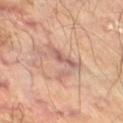Assessment:
This lesion was catalogued during total-body skin photography and was not selected for biopsy.
Background:
Cropped from a whole-body photographic skin survey; the tile spans about 15 mm. The lesion is located on the leg. A male patient, aged approximately 70. This is a cross-polarized tile.